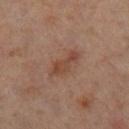Notes:
- follow-up · total-body-photography surveillance lesion; no biopsy
- image source · 15 mm crop, total-body photography
- image-analysis metrics · roughly 7 lightness units darker than nearby skin and a lesion-to-skin contrast of about 6.5 (normalized; higher = more distinct); a peripheral color-asymmetry measure near 0.5
- tile lighting · cross-polarized illumination
- patient · male, about 60 years old
- body site · the right lower leg
- lesion size · ≈4.5 mm